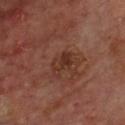Captured during whole-body skin photography for melanoma surveillance; the lesion was not biopsied.
From the chest.
A close-up tile cropped from a whole-body skin photograph, about 15 mm across.
The subject is a male aged around 65.